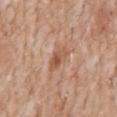Part of a total-body skin-imaging series; this lesion was reviewed on a skin check and was not flagged for biopsy. Captured under white-light illumination. From the chest. A 15 mm close-up extracted from a 3D total-body photography capture. An algorithmic analysis of the crop reported a lesion area of about 5 mm², an eccentricity of roughly 0.9, and a shape-asymmetry score of about 0.3 (0 = symmetric). The software also gave an average lesion color of about L≈55 a*≈21 b*≈32 (CIELAB) and a normalized border contrast of about 7. It also reported a lesion-detection confidence of about 100/100. The subject is a male in their 60s. Longest diameter approximately 3.5 mm.